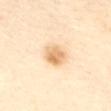{"biopsy_status": "not biopsied; imaged during a skin examination", "image": {"source": "total-body photography crop", "field_of_view_mm": 15}, "lesion_size": {"long_diameter_mm_approx": 3.0}, "lighting": "cross-polarized", "site": "abdomen", "patient": {"sex": "female", "age_approx": 65}}